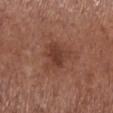Notes:
• notes — total-body-photography surveillance lesion; no biopsy
• body site — the left lower leg
• size — ≈4.5 mm
• subject — female, aged 53–57
• automated metrics — an area of roughly 9.5 mm²; about 8 CIELAB-L* units darker than the surrounding skin and a normalized lesion–skin contrast near 6.5; border irregularity of about 4 on a 0–10 scale, a within-lesion color-variation index near 3/10, and radial color variation of about 1; a classifier nevus-likeness of about 35/100 and a detector confidence of about 100 out of 100 that the crop contains a lesion
• acquisition — total-body-photography crop, ~15 mm field of view